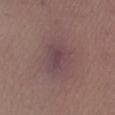lesion diameter: ~4 mm (longest diameter) | TBP lesion metrics: an area of roughly 8.5 mm² and a shape-asymmetry score of about 0.35 (0 = symmetric); an average lesion color of about L≈42 a*≈18 b*≈14 (CIELAB), roughly 6 lightness units darker than nearby skin, and a normalized border contrast of about 6.5; a border-irregularity rating of about 3.5/10, internal color variation of about 2.5 on a 0–10 scale, and peripheral color asymmetry of about 0.5; a nevus-likeness score of about 0/100 and a lesion-detection confidence of about 60/100 | image source: 15 mm crop, total-body photography | subject: female, aged 43–47 | location: the leg.